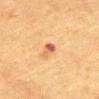Assessment: Recorded during total-body skin imaging; not selected for excision or biopsy. Image and clinical context: The lesion is located on the abdomen. The total-body-photography lesion software estimated a footprint of about 3 mm², an outline eccentricity of about 0.85 (0 = round, 1 = elongated), and two-axis asymmetry of about 0.35. And it measured a mean CIELAB color near L≈51 a*≈24 b*≈33, a lesion–skin lightness drop of about 11, and a normalized border contrast of about 8. The software also gave a border-irregularity rating of about 3/10, a within-lesion color-variation index near 5/10, and radial color variation of about 1. It also reported a detector confidence of about 100 out of 100 that the crop contains a lesion. A female subject, about 55 years old. The tile uses cross-polarized illumination. This image is a 15 mm lesion crop taken from a total-body photograph.